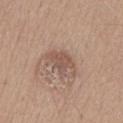| field | value |
|---|---|
| biopsy status | total-body-photography surveillance lesion; no biopsy |
| automated lesion analysis | a lesion area of about 6.5 mm²; a lesion color around L≈53 a*≈18 b*≈26 in CIELAB, a lesion–skin lightness drop of about 8, and a normalized lesion–skin contrast near 6; an automated nevus-likeness rating near 0 out of 100 and lesion-presence confidence of about 100/100 |
| illumination | white-light |
| imaging modality | ~15 mm tile from a whole-body skin photo |
| subject | male, roughly 75 years of age |
| location | the abdomen |
| diameter | about 3.5 mm |